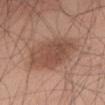{"biopsy_status": "not biopsied; imaged during a skin examination", "patient": {"sex": "male", "age_approx": 40}, "image": {"source": "total-body photography crop", "field_of_view_mm": 15}, "automated_metrics": {"area_mm2_approx": 25.0, "eccentricity": 0.8, "shape_asymmetry": 0.15, "cielab_L": 50, "cielab_a": 20, "cielab_b": 27, "vs_skin_contrast_norm": 7.0, "border_irregularity_0_10": 2.0, "color_variation_0_10": 3.5, "peripheral_color_asymmetry": 1.0, "nevus_likeness_0_100": 65}, "lighting": "white-light", "site": "abdomen"}